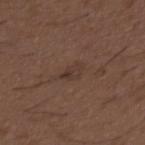Captured during whole-body skin photography for melanoma surveillance; the lesion was not biopsied. The total-body-photography lesion software estimated a mean CIELAB color near L≈35 a*≈15 b*≈22 and a lesion–skin lightness drop of about 6. It also reported a nevus-likeness score of about 0/100. Captured under white-light illumination. From the upper back. A 15 mm close-up extracted from a 3D total-body photography capture. A male patient aged approximately 50.